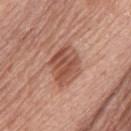Notes:
• notes · imaged on a skin check; not biopsied
• acquisition · ~15 mm tile from a whole-body skin photo
• lesion size · ≈4.5 mm
• site · the left thigh
• patient · female, aged 73–77
• illumination · white-light